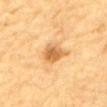  lesion_size:
    long_diameter_mm_approx: 3.5
  patient:
    sex: male
    age_approx: 85+
  image:
    source: total-body photography crop
    field_of_view_mm: 15
  lighting: cross-polarized
  site: abdomen
  automated_metrics:
    area_mm2_approx: 7.0
    eccentricity: 0.5
    border_irregularity_0_10: 3.5
    color_variation_0_10: 4.0
    peripheral_color_asymmetry: 1.0
    nevus_likeness_0_100: 80
    lesion_detection_confidence_0_100: 100